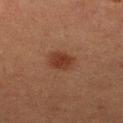- follow-up: imaged on a skin check; not biopsied
- automated lesion analysis: an area of roughly 6 mm² and an eccentricity of roughly 0.6; a lesion–skin lightness drop of about 8 and a normalized lesion–skin contrast near 8; lesion-presence confidence of about 100/100
- location: the right lower leg
- acquisition: ~15 mm crop, total-body skin-cancer survey
- illumination: cross-polarized
- subject: female, approximately 50 years of age
- lesion size: ≈3 mm